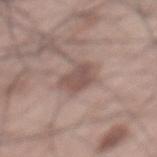Q: Was a biopsy performed?
A: total-body-photography surveillance lesion; no biopsy
Q: How large is the lesion?
A: ~4 mm (longest diameter)
Q: Lesion location?
A: the mid back
Q: What is the imaging modality?
A: total-body-photography crop, ~15 mm field of view
Q: Who is the patient?
A: male, aged around 70
Q: What lighting was used for the tile?
A: white-light